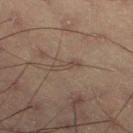Assessment:
Part of a total-body skin-imaging series; this lesion was reviewed on a skin check and was not flagged for biopsy.
Acquisition and patient details:
A roughly 15 mm field-of-view crop from a total-body skin photograph. A male patient, aged approximately 55. On the leg. The recorded lesion diameter is about 3 mm.